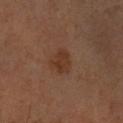Clinical impression: Part of a total-body skin-imaging series; this lesion was reviewed on a skin check and was not flagged for biopsy.